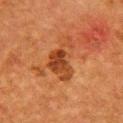Assessment:
This lesion was catalogued during total-body skin photography and was not selected for biopsy.
Image and clinical context:
The patient is a female approximately 50 years of age. The lesion's longest dimension is about 5.5 mm. A 15 mm close-up extracted from a 3D total-body photography capture. The lesion is located on the chest. The tile uses cross-polarized illumination.